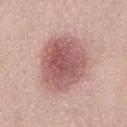Acquisition and patient details:
Located on the abdomen. A female subject, in their mid-60s. A close-up tile cropped from a whole-body skin photograph, about 15 mm across.